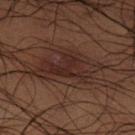• follow-up · imaged on a skin check; not biopsied
• illumination · cross-polarized
• image source · ~15 mm tile from a whole-body skin photo
• anatomic site · the left lower leg
• patient · male, approximately 50 years of age